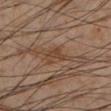Assessment:
This lesion was catalogued during total-body skin photography and was not selected for biopsy.
Image and clinical context:
From the left lower leg. A 15 mm crop from a total-body photograph taken for skin-cancer surveillance. The patient is a male aged approximately 55.